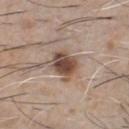The patient is a male about 60 years old.
Imaged with white-light lighting.
This image is a 15 mm lesion crop taken from a total-body photograph.
Automated image analysis of the tile measured a lesion area of about 10 mm².
From the chest.
Measured at roughly 4 mm in maximum diameter.